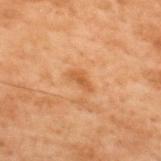{
  "biopsy_status": "not biopsied; imaged during a skin examination",
  "site": "upper back",
  "lesion_size": {
    "long_diameter_mm_approx": 3.0
  },
  "lighting": "cross-polarized",
  "image": {
    "source": "total-body photography crop",
    "field_of_view_mm": 15
  },
  "patient": {
    "sex": "male",
    "age_approx": 70
  }
}